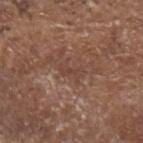  automated_metrics:
    cielab_L: 42
    cielab_a: 19
    cielab_b: 25
    vs_skin_darker_L: 6.0
    vs_skin_contrast_norm: 5.0
    lesion_detection_confidence_0_100: 60
  lesion_size:
    long_diameter_mm_approx: 2.5
  site: head or neck
  patient:
    sex: male
    age_approx: 80
  image:
    source: total-body photography crop
    field_of_view_mm: 15
  lighting: white-light